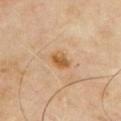workup = total-body-photography surveillance lesion; no biopsy
lighting = cross-polarized
image source = ~15 mm tile from a whole-body skin photo
lesion size = about 2.5 mm
body site = the chest
patient = male, in their mid- to late 60s